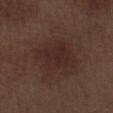Notes:
* biopsy status — imaged on a skin check; not biopsied
* acquisition — 15 mm crop, total-body photography
* anatomic site — the right thigh
* size — about 4 mm
* tile lighting — white-light illumination
* automated lesion analysis — a footprint of about 13 mm², a shape eccentricity near 0.5, and a symmetry-axis asymmetry near 0.2
* patient — male, in their 70s